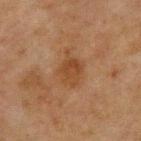Q: Was this lesion biopsied?
A: catalogued during a skin exam; not biopsied
Q: Where on the body is the lesion?
A: the chest
Q: What are the patient's age and sex?
A: male, about 65 years old
Q: What kind of image is this?
A: 15 mm crop, total-body photography